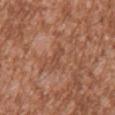No biopsy was performed on this lesion — it was imaged during a full skin examination and was not determined to be concerning. The tile uses white-light illumination. A male subject, about 45 years old. Approximately 3 mm at its widest. A 15 mm close-up tile from a total-body photography series done for melanoma screening. The total-body-photography lesion software estimated a footprint of about 3.5 mm². It also reported border irregularity of about 5 on a 0–10 scale, a within-lesion color-variation index near 0.5/10, and radial color variation of about 0. The lesion is located on the left upper arm.